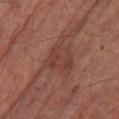<case>
<biopsy_status>not biopsied; imaged during a skin examination</biopsy_status>
<image>
  <source>total-body photography crop</source>
  <field_of_view_mm>15</field_of_view_mm>
</image>
<lesion_size>
  <long_diameter_mm_approx>4.0</long_diameter_mm_approx>
</lesion_size>
<site>leg</site>
<patient>
  <sex>male</sex>
  <age_approx>65</age_approx>
</patient>
</case>